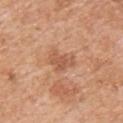Part of a total-body skin-imaging series; this lesion was reviewed on a skin check and was not flagged for biopsy.
A male subject aged 58 to 62.
This image is a 15 mm lesion crop taken from a total-body photograph.
From the upper back.